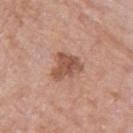Part of a total-body skin-imaging series; this lesion was reviewed on a skin check and was not flagged for biopsy. The lesion is on the right upper arm. This is a white-light tile. A roughly 15 mm field-of-view crop from a total-body skin photograph. The subject is a female aged around 85 or older.